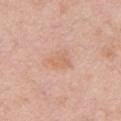Part of a total-body skin-imaging series; this lesion was reviewed on a skin check and was not flagged for biopsy. The lesion is on the chest. The tile uses white-light illumination. A male patient, roughly 50 years of age. A roughly 15 mm field-of-view crop from a total-body skin photograph.